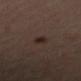Assessment:
Captured during whole-body skin photography for melanoma surveillance; the lesion was not biopsied.
Acquisition and patient details:
From the right thigh. Captured under cross-polarized illumination. Longest diameter approximately 2.5 mm. A male subject, aged approximately 65. An algorithmic analysis of the crop reported a lesion area of about 3.5 mm², an eccentricity of roughly 0.75, and a symmetry-axis asymmetry near 0.3. The software also gave an average lesion color of about L≈25 a*≈13 b*≈19 (CIELAB) and a lesion-to-skin contrast of about 8 (normalized; higher = more distinct). The software also gave border irregularity of about 2.5 on a 0–10 scale, a color-variation rating of about 2/10, and peripheral color asymmetry of about 0.5. A lesion tile, about 15 mm wide, cut from a 3D total-body photograph.